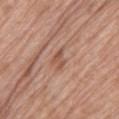Imaged during a routine full-body skin examination; the lesion was not biopsied and no histopathology is available. Located on the left thigh. The patient is a female roughly 75 years of age. Imaged with white-light lighting. The lesion-visualizer software estimated roughly 8 lightness units darker than nearby skin. A roughly 15 mm field-of-view crop from a total-body skin photograph.